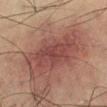Notes:
• follow-up — no biopsy performed (imaged during a skin exam)